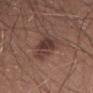No biopsy was performed on this lesion — it was imaged during a full skin examination and was not determined to be concerning. From the leg. A male patient aged 28–32. Measured at roughly 6 mm in maximum diameter. The tile uses white-light illumination. A lesion tile, about 15 mm wide, cut from a 3D total-body photograph.